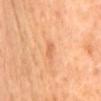follow-up: catalogued during a skin exam; not biopsied | diameter: ~2.5 mm (longest diameter) | acquisition: total-body-photography crop, ~15 mm field of view | body site: the mid back | patient: female, in their 50s | lighting: cross-polarized illumination.